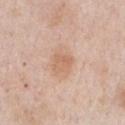A 15 mm close-up tile from a total-body photography series done for melanoma screening. A male subject, in their 60s. The lesion is on the chest. Longest diameter approximately 2.5 mm.